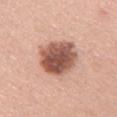Recorded during total-body skin imaging; not selected for excision or biopsy. This is a white-light tile. A 15 mm crop from a total-body photograph taken for skin-cancer surveillance. A female patient, in their mid- to late 20s. About 5 mm across. Located on the upper back.